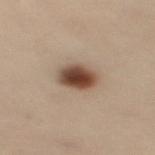Case summary:
• notes — no biopsy performed (imaged during a skin exam)
• automated lesion analysis — a border-irregularity rating of about 1.5/10, internal color variation of about 6 on a 0–10 scale, and radial color variation of about 1.5; a nevus-likeness score of about 100/100 and a lesion-detection confidence of about 100/100
• anatomic site — the right thigh
• imaging modality — ~15 mm crop, total-body skin-cancer survey
• subject — female, aged 48–52
• illumination — cross-polarized
• lesion diameter — ≈4 mm